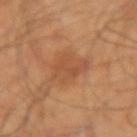Impression: The lesion was photographed on a routine skin check and not biopsied; there is no pathology result. Context: A lesion tile, about 15 mm wide, cut from a 3D total-body photograph. Longest diameter approximately 4 mm. Imaged with cross-polarized lighting. Located on the right upper arm. The patient is a male about 55 years old. Automated image analysis of the tile measured an area of roughly 10 mm² and an outline eccentricity of about 0.6 (0 = round, 1 = elongated). And it measured a normalized border contrast of about 5.